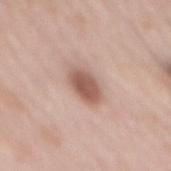Background: This is a white-light tile. About 4 mm across. Automated image analysis of the tile measured an average lesion color of about L≈56 a*≈21 b*≈26 (CIELAB) and a lesion-to-skin contrast of about 9.5 (normalized; higher = more distinct). A female patient approximately 65 years of age. On the mid back. A 15 mm crop from a total-body photograph taken for skin-cancer surveillance.